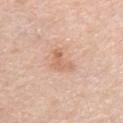| feature | finding |
|---|---|
| biopsy status | catalogued during a skin exam; not biopsied |
| lesion diameter | ~3.5 mm (longest diameter) |
| location | the right upper arm |
| subject | female, approximately 65 years of age |
| lighting | white-light illumination |
| acquisition | ~15 mm crop, total-body skin-cancer survey |
| TBP lesion metrics | a lesion color around L≈65 a*≈21 b*≈32 in CIELAB; a border-irregularity index near 5/10, internal color variation of about 3.5 on a 0–10 scale, and radial color variation of about 1.5 |